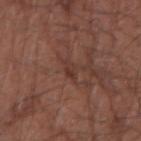Captured during whole-body skin photography for melanoma surveillance; the lesion was not biopsied.